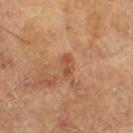Assessment:
Imaged during a routine full-body skin examination; the lesion was not biopsied and no histopathology is available.
Context:
A male patient aged 63 to 67. This is a cross-polarized tile. Automated tile analysis of the lesion measured a border-irregularity rating of about 3.5/10, internal color variation of about 0 on a 0–10 scale, and radial color variation of about 0. It also reported a nevus-likeness score of about 0/100 and a detector confidence of about 100 out of 100 that the crop contains a lesion. The lesion is located on the right lower leg. Measured at roughly 2.5 mm in maximum diameter. A region of skin cropped from a whole-body photographic capture, roughly 15 mm wide.